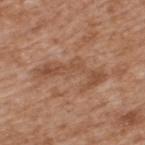Impression:
Recorded during total-body skin imaging; not selected for excision or biopsy.
Background:
A male subject approximately 65 years of age. From the upper back. Imaged with white-light lighting. The total-body-photography lesion software estimated a footprint of about 14 mm², an outline eccentricity of about 0.95 (0 = round, 1 = elongated), and two-axis asymmetry of about 0.6. The analysis additionally found border irregularity of about 9.5 on a 0–10 scale, a color-variation rating of about 3/10, and radial color variation of about 1. A roughly 15 mm field-of-view crop from a total-body skin photograph.